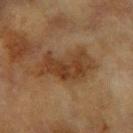Clinical impression: Captured during whole-body skin photography for melanoma surveillance; the lesion was not biopsied. Clinical summary: A region of skin cropped from a whole-body photographic capture, roughly 15 mm wide. The recorded lesion diameter is about 7 mm. Captured under cross-polarized illumination. From the right forearm. A female subject aged approximately 60. The lesion-visualizer software estimated a footprint of about 18 mm², a shape eccentricity near 0.85, and a shape-asymmetry score of about 0.35 (0 = symmetric). The software also gave a lesion color around L≈36 a*≈18 b*≈31 in CIELAB and about 9 CIELAB-L* units darker than the surrounding skin. The analysis additionally found border irregularity of about 4.5 on a 0–10 scale, internal color variation of about 4 on a 0–10 scale, and peripheral color asymmetry of about 1.5.